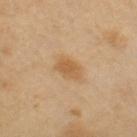Assessment:
The lesion was tiled from a total-body skin photograph and was not biopsied.
Context:
An algorithmic analysis of the crop reported a shape eccentricity near 0.8 and a shape-asymmetry score of about 0.25 (0 = symmetric). The analysis additionally found an average lesion color of about L≈56 a*≈18 b*≈38 (CIELAB) and a lesion-to-skin contrast of about 7 (normalized; higher = more distinct). The software also gave a border-irregularity index near 2/10 and peripheral color asymmetry of about 0.5. Approximately 3 mm at its widest. A female subject aged 48–52. A 15 mm close-up extracted from a 3D total-body photography capture. From the right upper arm.